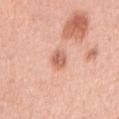The lesion was tiled from a total-body skin photograph and was not biopsied. A female subject, in their 60s. Captured under white-light illumination. The lesion is on the right upper arm. A 15 mm close-up extracted from a 3D total-body photography capture. Automated tile analysis of the lesion measured a border-irregularity rating of about 2/10, a color-variation rating of about 4/10, and peripheral color asymmetry of about 1.5. About 2.5 mm across.